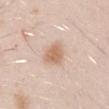{
  "automated_metrics": {
    "border_irregularity_0_10": 2.0,
    "color_variation_0_10": 2.0,
    "peripheral_color_asymmetry": 0.5,
    "nevus_likeness_0_100": 95
  },
  "patient": {
    "sex": "male",
    "age_approx": 30
  },
  "image": {
    "source": "total-body photography crop",
    "field_of_view_mm": 15
  },
  "lesion_size": {
    "long_diameter_mm_approx": 3.0
  },
  "site": "left upper arm",
  "lighting": "white-light"
}